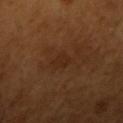- biopsy status — imaged on a skin check; not biopsied
- anatomic site — the right upper arm
- patient — male, aged 63 to 67
- diameter — about 2.5 mm
- lighting — cross-polarized illumination
- imaging modality — ~15 mm tile from a whole-body skin photo